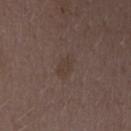Imaged during a routine full-body skin examination; the lesion was not biopsied and no histopathology is available. Longest diameter approximately 3 mm. The patient is a female roughly 30 years of age. The tile uses white-light illumination. This image is a 15 mm lesion crop taken from a total-body photograph. On the left forearm.